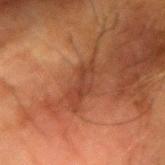{
  "biopsy_status": "not biopsied; imaged during a skin examination",
  "image": {
    "source": "total-body photography crop",
    "field_of_view_mm": 15
  },
  "lesion_size": {
    "long_diameter_mm_approx": 5.0
  },
  "lighting": "cross-polarized",
  "patient": {
    "sex": "male",
    "age_approx": 65
  },
  "site": "left forearm"
}